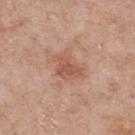Assessment:
The lesion was photographed on a routine skin check and not biopsied; there is no pathology result.
Background:
An algorithmic analysis of the crop reported an automated nevus-likeness rating near 0 out of 100. The tile uses white-light illumination. A male subject, in their mid- to late 50s. A lesion tile, about 15 mm wide, cut from a 3D total-body photograph. On the upper back.